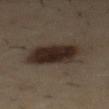Part of a total-body skin-imaging series; this lesion was reviewed on a skin check and was not flagged for biopsy.
This is a cross-polarized tile.
The lesion is on the mid back.
A male subject approximately 55 years of age.
A close-up tile cropped from a whole-body skin photograph, about 15 mm across.